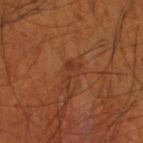The lesion-visualizer software estimated a lesion area of about 3.5 mm² and an eccentricity of roughly 0.85. The analysis additionally found a border-irregularity index near 7/10, a color-variation rating of about 0.5/10, and a peripheral color-asymmetry measure near 0. And it measured a classifier nevus-likeness of about 0/100 and lesion-presence confidence of about 95/100. A male subject aged approximately 55. Approximately 3 mm at its widest. This is a cross-polarized tile. The lesion is on the right thigh. This image is a 15 mm lesion crop taken from a total-body photograph.